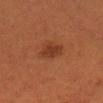Recorded during total-body skin imaging; not selected for excision or biopsy. The tile uses cross-polarized illumination. On the head or neck. The patient is a female about 40 years old. A 15 mm close-up extracted from a 3D total-body photography capture. The lesion's longest dimension is about 2.5 mm. Automated image analysis of the tile measured an area of roughly 4.5 mm², an eccentricity of roughly 0.75, and two-axis asymmetry of about 0.2. The software also gave a border-irregularity index near 2/10, a within-lesion color-variation index near 1.5/10, and radial color variation of about 0.5. And it measured a classifier nevus-likeness of about 70/100 and a detector confidence of about 100 out of 100 that the crop contains a lesion.